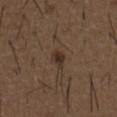This lesion was catalogued during total-body skin photography and was not selected for biopsy. On the front of the torso. The patient is a male in their 50s. Cropped from a total-body skin-imaging series; the visible field is about 15 mm. The lesion's longest dimension is about 2.5 mm. Captured under white-light illumination.